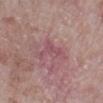The lesion was tiled from a total-body skin photograph and was not biopsied.
Imaged with white-light lighting.
Located on the leg.
The patient is a female approximately 70 years of age.
A 15 mm close-up tile from a total-body photography series done for melanoma screening.
About 4.5 mm across.